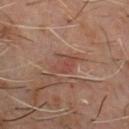Imaged during a routine full-body skin examination; the lesion was not biopsied and no histopathology is available. A male patient, aged 58 to 62. Cropped from a whole-body photographic skin survey; the tile spans about 15 mm. The lesion is on the chest. This is a cross-polarized tile. The lesion-visualizer software estimated a normalized lesion–skin contrast near 5.